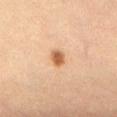Q: Was a biopsy performed?
A: no biopsy performed (imaged during a skin exam)
Q: What are the patient's age and sex?
A: female, aged approximately 40
Q: What is the anatomic site?
A: the lower back
Q: What lighting was used for the tile?
A: cross-polarized
Q: Lesion size?
A: ≈2.5 mm
Q: What is the imaging modality?
A: ~15 mm crop, total-body skin-cancer survey